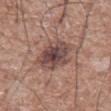Assessment: The lesion was photographed on a routine skin check and not biopsied; there is no pathology result. Context: A 15 mm crop from a total-body photograph taken for skin-cancer surveillance. The total-body-photography lesion software estimated roughly 12 lightness units darker than nearby skin and a normalized lesion–skin contrast near 9.5. The analysis additionally found border irregularity of about 2.5 on a 0–10 scale, a color-variation rating of about 6.5/10, and radial color variation of about 2. This is a white-light tile. Measured at roughly 4.5 mm in maximum diameter. On the front of the torso. The patient is a male approximately 75 years of age.